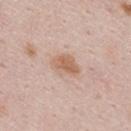Imaged during a routine full-body skin examination; the lesion was not biopsied and no histopathology is available.
A male patient, aged 23 to 27.
The lesion is located on the back.
A region of skin cropped from a whole-body photographic capture, roughly 15 mm wide.
The tile uses white-light illumination.